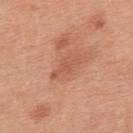The lesion was photographed on a routine skin check and not biopsied; there is no pathology result.
A male patient approximately 70 years of age.
Cropped from a whole-body photographic skin survey; the tile spans about 15 mm.
The tile uses white-light illumination.
The lesion's longest dimension is about 4 mm.
Automated tile analysis of the lesion measured a lesion color around L≈56 a*≈25 b*≈33 in CIELAB and a normalized border contrast of about 5. The analysis additionally found border irregularity of about 5.5 on a 0–10 scale, a color-variation rating of about 1/10, and radial color variation of about 0.5.
From the upper back.